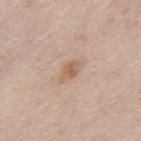biopsy status = catalogued during a skin exam; not biopsied | location = the upper back | acquisition = 15 mm crop, total-body photography | subject = male, approximately 40 years of age.